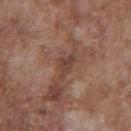<lesion>
  <biopsy_status>not biopsied; imaged during a skin examination</biopsy_status>
  <lesion_size>
    <long_diameter_mm_approx>3.5</long_diameter_mm_approx>
  </lesion_size>
  <patient>
    <sex>male</sex>
    <age_approx>75</age_approx>
  </patient>
  <image>
    <source>total-body photography crop</source>
    <field_of_view_mm>15</field_of_view_mm>
  </image>
  <lighting>white-light</lighting>
  <site>chest</site>
  <automated_metrics>
    <area_mm2_approx>4.0</area_mm2_approx>
    <eccentricity>0.85</eccentricity>
    <shape_asymmetry>0.45</shape_asymmetry>
    <border_irregularity_0_10>5.0</border_irregularity_0_10>
    <color_variation_0_10>1.5</color_variation_0_10>
    <peripheral_color_asymmetry>0.5</peripheral_color_asymmetry>
    <lesion_detection_confidence_0_100>55</lesion_detection_confidence_0_100>
  </automated_metrics>
</lesion>